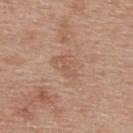| key | value |
|---|---|
| biopsy status | total-body-photography surveillance lesion; no biopsy |
| site | the back |
| patient | female, approximately 40 years of age |
| lighting | white-light illumination |
| size | about 3.5 mm |
| imaging modality | 15 mm crop, total-body photography |
| image-analysis metrics | a lesion area of about 4 mm² and two-axis asymmetry of about 0.45; a lesion–skin lightness drop of about 6 and a lesion-to-skin contrast of about 4.5 (normalized; higher = more distinct); a border-irregularity rating of about 5/10, a within-lesion color-variation index near 1/10, and peripheral color asymmetry of about 0.5 |